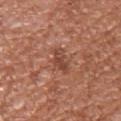{"biopsy_status": "not biopsied; imaged during a skin examination", "image": {"source": "total-body photography crop", "field_of_view_mm": 15}, "site": "left upper arm", "patient": {"sex": "female", "age_approx": 40}, "lighting": "white-light"}